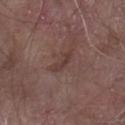Case summary:
* follow-up — no biopsy performed (imaged during a skin exam)
* subject — male, aged 63–67
* location — the right forearm
* lesion size — ~3 mm (longest diameter)
* image source — 15 mm crop, total-body photography
* tile lighting — white-light illumination
* automated metrics — a footprint of about 3.5 mm² and an outline eccentricity of about 0.9 (0 = round, 1 = elongated)